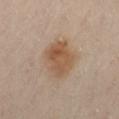The lesion was tiled from a total-body skin photograph and was not biopsied. The total-body-photography lesion software estimated two-axis asymmetry of about 0.15. Located on the front of the torso. Longest diameter approximately 5 mm. Captured under cross-polarized illumination. A roughly 15 mm field-of-view crop from a total-body skin photograph. A female patient aged approximately 40.